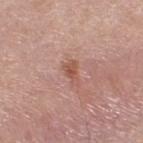Case summary:
* workup — catalogued during a skin exam; not biopsied
* subject — male, aged around 45
* image — ~15 mm crop, total-body skin-cancer survey
* lesion size — ~3 mm (longest diameter)
* TBP lesion metrics — a footprint of about 3.5 mm² and an outline eccentricity of about 0.8 (0 = round, 1 = elongated); an average lesion color of about L≈53 a*≈23 b*≈28 (CIELAB), about 9 CIELAB-L* units darker than the surrounding skin, and a lesion-to-skin contrast of about 7 (normalized; higher = more distinct)
* lighting — white-light
* anatomic site — the upper back